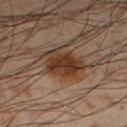| field | value |
|---|---|
| tile lighting | cross-polarized |
| subject | male, aged around 55 |
| anatomic site | the left lower leg |
| image source | ~15 mm crop, total-body skin-cancer survey |
| size | ≈5 mm |
| image-analysis metrics | a lesion area of about 12 mm², a shape eccentricity near 0.75, and two-axis asymmetry of about 0.2; a lesion–skin lightness drop of about 13 and a lesion-to-skin contrast of about 11 (normalized; higher = more distinct); a lesion-detection confidence of about 100/100 |